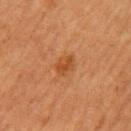biopsy status=catalogued during a skin exam; not biopsied | imaging modality=15 mm crop, total-body photography | subject=female, about 55 years old | lighting=cross-polarized | automated lesion analysis=an area of roughly 4.5 mm² and two-axis asymmetry of about 0.2 | lesion size=~2.5 mm (longest diameter) | site=the left upper arm.